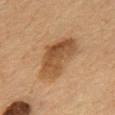Captured during whole-body skin photography for melanoma surveillance; the lesion was not biopsied. A male subject, about 55 years old. From the back. This image is a 15 mm lesion crop taken from a total-body photograph.